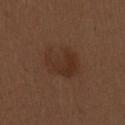A roughly 15 mm field-of-view crop from a total-body skin photograph. The total-body-photography lesion software estimated a footprint of about 13 mm², an eccentricity of roughly 0.6, and a symmetry-axis asymmetry near 0.25. The analysis additionally found a mean CIELAB color near L≈31 a*≈18 b*≈26, about 6 CIELAB-L* units darker than the surrounding skin, and a normalized border contrast of about 6. And it measured a classifier nevus-likeness of about 55/100. Located on the left upper arm. The patient is a female aged approximately 30. Measured at roughly 4.5 mm in maximum diameter.